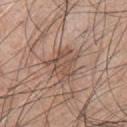<tbp_lesion>
<biopsy_status>not biopsied; imaged during a skin examination</biopsy_status>
<patient>
  <sex>male</sex>
  <age_approx>45</age_approx>
</patient>
<automated_metrics>
  <vs_skin_darker_L>8.0</vs_skin_darker_L>
  <vs_skin_contrast_norm>6.0</vs_skin_contrast_norm>
  <border_irregularity_0_10>7.0</border_irregularity_0_10>
  <color_variation_0_10>3.0</color_variation_0_10>
  <peripheral_color_asymmetry>1.0</peripheral_color_asymmetry>
</automated_metrics>
<site>chest</site>
<image>
  <source>total-body photography crop</source>
  <field_of_view_mm>15</field_of_view_mm>
</image>
<lesion_size>
  <long_diameter_mm_approx>3.5</long_diameter_mm_approx>
</lesion_size>
</tbp_lesion>